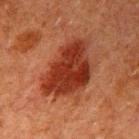Clinical summary:
About 8 mm across. This is a cross-polarized tile. A male patient aged around 60. Cropped from a total-body skin-imaging series; the visible field is about 15 mm. The lesion is on the right upper arm.